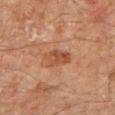  biopsy_status: not biopsied; imaged during a skin examination
  site: right upper arm
  patient:
    sex: male
    age_approx: 45
  image:
    source: total-body photography crop
    field_of_view_mm: 15
  lesion_size:
    long_diameter_mm_approx: 3.0
  lighting: cross-polarized